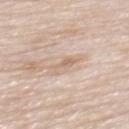Notes:
- follow-up: catalogued during a skin exam; not biopsied
- image-analysis metrics: a border-irregularity rating of about 5/10, internal color variation of about 1 on a 0–10 scale, and a peripheral color-asymmetry measure near 0.5; a nevus-likeness score of about 0/100
- image: 15 mm crop, total-body photography
- patient: male, in their mid- to late 80s or older
- lesion size: ≈2.5 mm
- body site: the upper back
- tile lighting: white-light